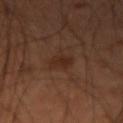<case>
  <image>
    <source>total-body photography crop</source>
    <field_of_view_mm>15</field_of_view_mm>
  </image>
  <patient>
    <sex>male</sex>
    <age_approx>50</age_approx>
  </patient>
  <site>arm</site>
</case>